This is a cross-polarized tile; Automated tile analysis of the lesion measured internal color variation of about 5 on a 0–10 scale and peripheral color asymmetry of about 2; about 5.5 mm across; the patient is a male in their mid-40s; cropped from a total-body skin-imaging series; the visible field is about 15 mm; the lesion is located on the left thigh: 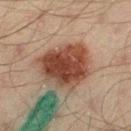Biopsy histopathology demonstrated a dysplastic (Clark) nevus — a benign lesion.